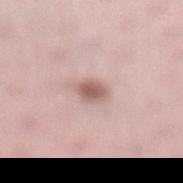Recorded during total-body skin imaging; not selected for excision or biopsy. The total-body-photography lesion software estimated an area of roughly 4.5 mm² and a shape-asymmetry score of about 0.15 (0 = symmetric). A 15 mm crop from a total-body photograph taken for skin-cancer surveillance. A female patient, in their mid-30s. On the left lower leg. Captured under white-light illumination.